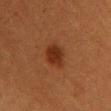workup=total-body-photography surveillance lesion; no biopsy
location=the chest
subject=female, approximately 30 years of age
size=~3 mm (longest diameter)
acquisition=15 mm crop, total-body photography